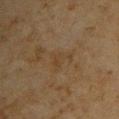Case summary:
• biopsy status: no biopsy performed (imaged during a skin exam)
• acquisition: ~15 mm tile from a whole-body skin photo
• patient: male, roughly 45 years of age
• body site: the upper back